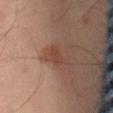follow-up — catalogued during a skin exam; not biopsied
imaging modality — total-body-photography crop, ~15 mm field of view
patient — male, aged 63 to 67
automated metrics — a lesion color around L≈41 a*≈20 b*≈26 in CIELAB and a normalized border contrast of about 6; a color-variation rating of about 1.5/10 and peripheral color asymmetry of about 0.5
tile lighting — cross-polarized illumination
site — the right thigh